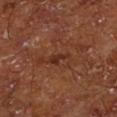  biopsy_status: not biopsied; imaged during a skin examination
  lighting: cross-polarized
  image:
    source: total-body photography crop
    field_of_view_mm: 15
  site: left lower leg
  patient:
    sex: male
    age_approx: 65